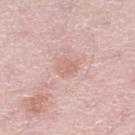The lesion was photographed on a routine skin check and not biopsied; there is no pathology result. The lesion's longest dimension is about 2.5 mm. This is a white-light tile. Automated image analysis of the tile measured a mean CIELAB color near L≈66 a*≈21 b*≈26 and a normalized lesion–skin contrast near 5. It also reported a border-irregularity rating of about 2/10, a color-variation rating of about 2/10, and a peripheral color-asymmetry measure near 1. A male patient aged 48–52. The lesion is located on the left lower leg. A region of skin cropped from a whole-body photographic capture, roughly 15 mm wide.